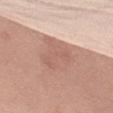Q: Is there a histopathology result?
A: total-body-photography surveillance lesion; no biopsy
Q: Illumination type?
A: white-light illumination
Q: What is the imaging modality?
A: total-body-photography crop, ~15 mm field of view
Q: What did automated image analysis measure?
A: an average lesion color of about L≈60 a*≈21 b*≈26 (CIELAB), about 9 CIELAB-L* units darker than the surrounding skin, and a lesion-to-skin contrast of about 5.5 (normalized; higher = more distinct); border irregularity of about 3.5 on a 0–10 scale and a color-variation rating of about 2/10; an automated nevus-likeness rating near 0 out of 100 and lesion-presence confidence of about 55/100
Q: Where on the body is the lesion?
A: the left thigh
Q: How large is the lesion?
A: about 5 mm
Q: Who is the patient?
A: female, in their mid-50s